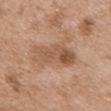Impression: This lesion was catalogued during total-body skin photography and was not selected for biopsy. Image and clinical context: A roughly 15 mm field-of-view crop from a total-body skin photograph. The lesion's longest dimension is about 6 mm. The subject is a male aged 48 to 52. On the chest. An algorithmic analysis of the crop reported a shape-asymmetry score of about 0.25 (0 = symmetric). The software also gave a mean CIELAB color near L≈54 a*≈20 b*≈32 and a normalized lesion–skin contrast near 6.5. It also reported a classifier nevus-likeness of about 0/100 and a lesion-detection confidence of about 100/100. The tile uses white-light illumination.